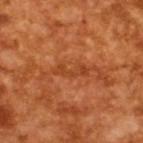{
  "biopsy_status": "not biopsied; imaged during a skin examination",
  "image": {
    "source": "total-body photography crop",
    "field_of_view_mm": 15
  },
  "lighting": "cross-polarized",
  "patient": {
    "sex": "male",
    "age_approx": 65
  },
  "lesion_size": {
    "long_diameter_mm_approx": 4.0
  }
}